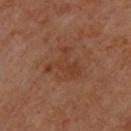Findings:
* notes · catalogued during a skin exam; not biopsied
* anatomic site · the upper back
* imaging modality · 15 mm crop, total-body photography
* patient · aged approximately 65
* lighting · cross-polarized illumination